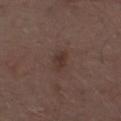Imaged during a routine full-body skin examination; the lesion was not biopsied and no histopathology is available. A close-up tile cropped from a whole-body skin photograph, about 15 mm across. Approximately 2.5 mm at its widest. The subject is a male approximately 70 years of age. On the left lower leg.